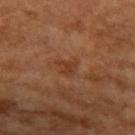No biopsy was performed on this lesion — it was imaged during a full skin examination and was not determined to be concerning.
The lesion is located on the left upper arm.
Cropped from a whole-body photographic skin survey; the tile spans about 15 mm.
Longest diameter approximately 2.5 mm.
This is a cross-polarized tile.
A male patient about 55 years old.